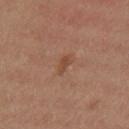Q: Is there a histopathology result?
A: imaged on a skin check; not biopsied
Q: What is the imaging modality?
A: ~15 mm tile from a whole-body skin photo
Q: Where on the body is the lesion?
A: the mid back
Q: What did automated image analysis measure?
A: a lesion color around L≈47 a*≈22 b*≈31 in CIELAB and roughly 8 lightness units darker than nearby skin; a lesion-detection confidence of about 100/100
Q: What lighting was used for the tile?
A: white-light illumination
Q: What is the lesion's diameter?
A: ~2.5 mm (longest diameter)
Q: Who is the patient?
A: female, aged approximately 30